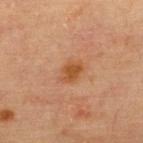Notes:
- notes: total-body-photography surveillance lesion; no biopsy
- subject: female, in their mid-60s
- acquisition: ~15 mm crop, total-body skin-cancer survey
- anatomic site: the back